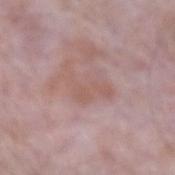biopsy status: total-body-photography surveillance lesion; no biopsy | location: the arm | lesion diameter: ~5 mm (longest diameter) | subject: male, approximately 65 years of age | image source: total-body-photography crop, ~15 mm field of view | TBP lesion metrics: an area of roughly 7 mm², a shape eccentricity near 0.85, and a shape-asymmetry score of about 0.7 (0 = symmetric); a lesion color around L≈56 a*≈20 b*≈23 in CIELAB and a normalized border contrast of about 5; a border-irregularity index near 9.5/10 and a peripheral color-asymmetry measure near 0.5 | lighting: white-light illumination.